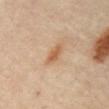The subject is a male in their mid-60s. Longest diameter approximately 3 mm. Imaged with cross-polarized lighting. The lesion is located on the front of the torso. Cropped from a whole-body photographic skin survey; the tile spans about 15 mm. The total-body-photography lesion software estimated an average lesion color of about L≈56 a*≈20 b*≈35 (CIELAB), roughly 9 lightness units darker than nearby skin, and a lesion-to-skin contrast of about 7 (normalized; higher = more distinct). It also reported a border-irregularity rating of about 3/10, a within-lesion color-variation index near 1.5/10, and peripheral color asymmetry of about 0.5. And it measured a classifier nevus-likeness of about 75/100 and a lesion-detection confidence of about 100/100.